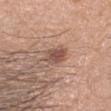Clinical impression: No biopsy was performed on this lesion — it was imaged during a full skin examination and was not determined to be concerning. Image and clinical context: Longest diameter approximately 3 mm. The lesion is on the head or neck. A male subject approximately 50 years of age. Cropped from a whole-body photographic skin survey; the tile spans about 15 mm. The lesion-visualizer software estimated an area of roughly 6 mm², an eccentricity of roughly 0.5, and two-axis asymmetry of about 0.15. The analysis additionally found a border-irregularity rating of about 1.5/10, a color-variation rating of about 3.5/10, and radial color variation of about 1.